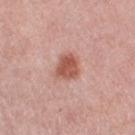notes = imaged on a skin check; not biopsied | TBP lesion metrics = a mean CIELAB color near L≈55 a*≈26 b*≈28 and about 13 CIELAB-L* units darker than the surrounding skin; a border-irregularity rating of about 2.5/10; a nevus-likeness score of about 100/100 and lesion-presence confidence of about 100/100 | location = the left thigh | subject = female, aged around 40 | lighting = white-light | image source = ~15 mm crop, total-body skin-cancer survey.